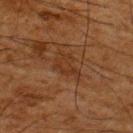Clinical impression: Captured during whole-body skin photography for melanoma surveillance; the lesion was not biopsied. Context: Cropped from a whole-body photographic skin survey; the tile spans about 15 mm. A male subject aged around 65. On the upper back. An algorithmic analysis of the crop reported a footprint of about 6 mm², an eccentricity of roughly 0.7, and a shape-asymmetry score of about 0.45 (0 = symmetric). And it measured a classifier nevus-likeness of about 0/100 and lesion-presence confidence of about 80/100. The tile uses cross-polarized illumination. Longest diameter approximately 3.5 mm.